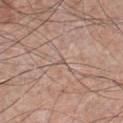location: the chest | imaging modality: 15 mm crop, total-body photography | TBP lesion metrics: an area of roughly 1 mm², a shape eccentricity near 0.75, and a shape-asymmetry score of about 0.3 (0 = symmetric) | patient: male, approximately 55 years of age | lesion diameter: about 1 mm.